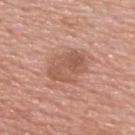Notes:
* biopsy status · no biopsy performed (imaged during a skin exam)
* patient · male, in their 60s
* body site · the upper back
* acquisition · total-body-photography crop, ~15 mm field of view